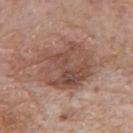notes: no biopsy performed (imaged during a skin exam)
automated lesion analysis: an eccentricity of roughly 0.6 and a shape-asymmetry score of about 0.2 (0 = symmetric); a border-irregularity index near 3.5/10, a within-lesion color-variation index near 6/10, and a peripheral color-asymmetry measure near 2
lesion size: ≈6.5 mm
anatomic site: the mid back
patient: male, about 75 years old
image source: total-body-photography crop, ~15 mm field of view